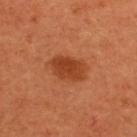No biopsy was performed on this lesion — it was imaged during a full skin examination and was not determined to be concerning.
A region of skin cropped from a whole-body photographic capture, roughly 15 mm wide.
The tile uses cross-polarized illumination.
A female patient, aged 48–52.